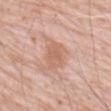Clinical impression:
This lesion was catalogued during total-body skin photography and was not selected for biopsy.
Image and clinical context:
The lesion is on the abdomen. An algorithmic analysis of the crop reported a lesion color around L≈62 a*≈22 b*≈30 in CIELAB and a normalized border contrast of about 5.5. Imaged with white-light lighting. A male patient approximately 80 years of age. A roughly 15 mm field-of-view crop from a total-body skin photograph.